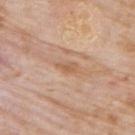- follow-up — imaged on a skin check; not biopsied
- illumination — white-light illumination
- acquisition — 15 mm crop, total-body photography
- automated metrics — a border-irregularity rating of about 3.5/10, a color-variation rating of about 0.5/10, and radial color variation of about 0
- anatomic site — the right upper arm
- patient — male, in their mid-70s